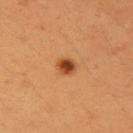| feature | finding |
|---|---|
| biopsy status | catalogued during a skin exam; not biopsied |
| subject | female, aged 38 to 42 |
| body site | the right upper arm |
| size | ~2 mm (longest diameter) |
| TBP lesion metrics | border irregularity of about 1.5 on a 0–10 scale, a color-variation rating of about 3.5/10, and a peripheral color-asymmetry measure near 1; an automated nevus-likeness rating near 100 out of 100 and lesion-presence confidence of about 100/100 |
| illumination | cross-polarized illumination |
| imaging modality | ~15 mm crop, total-body skin-cancer survey |